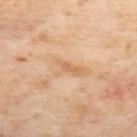biopsy_status: not biopsied; imaged during a skin examination
image:
  source: total-body photography crop
  field_of_view_mm: 15
patient:
  sex: female
  age_approx: 60
site: upper back
automated_metrics:
  area_mm2_approx: 7.5
  eccentricity: 0.9
  shape_asymmetry: 0.4
  nevus_likeness_0_100: 0
  lesion_detection_confidence_0_100: 100
lighting: cross-polarized